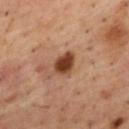* automated lesion analysis: a footprint of about 6 mm²; border irregularity of about 2 on a 0–10 scale; a nevus-likeness score of about 100/100 and a lesion-detection confidence of about 100/100
* subject: male, aged 53 to 57
* tile lighting: cross-polarized
* lesion diameter: ≈3 mm
* imaging modality: total-body-photography crop, ~15 mm field of view
* body site: the upper back